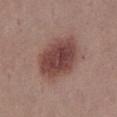Q: Was this lesion biopsied?
A: imaged on a skin check; not biopsied
Q: What is the lesion's diameter?
A: about 6 mm
Q: What is the anatomic site?
A: the abdomen
Q: How was this image acquired?
A: ~15 mm crop, total-body skin-cancer survey
Q: Patient demographics?
A: female, roughly 50 years of age